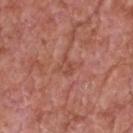Assessment: Imaged during a routine full-body skin examination; the lesion was not biopsied and no histopathology is available. Acquisition and patient details: A male subject roughly 60 years of age. Automated image analysis of the tile measured a footprint of about 2.5 mm² and two-axis asymmetry of about 0.5. And it measured a border-irregularity rating of about 5/10, internal color variation of about 1 on a 0–10 scale, and a peripheral color-asymmetry measure near 0.5. It also reported an automated nevus-likeness rating near 0 out of 100 and lesion-presence confidence of about 100/100. Imaged with white-light lighting. Cropped from a whole-body photographic skin survey; the tile spans about 15 mm. The lesion's longest dimension is about 2.5 mm. The lesion is on the head or neck.